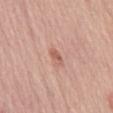Part of a total-body skin-imaging series; this lesion was reviewed on a skin check and was not flagged for biopsy. A female patient, in their mid- to late 60s. The tile uses white-light illumination. On the back. A lesion tile, about 15 mm wide, cut from a 3D total-body photograph. The lesion-visualizer software estimated a lesion area of about 2.5 mm² and a shape-asymmetry score of about 0.3 (0 = symmetric). The analysis additionally found a border-irregularity index near 3/10, a color-variation rating of about 0/10, and a peripheral color-asymmetry measure near 0.